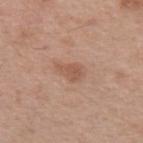Assessment: Captured during whole-body skin photography for melanoma surveillance; the lesion was not biopsied. Acquisition and patient details: Imaged with white-light lighting. A male subject, approximately 45 years of age. The lesion-visualizer software estimated a lesion area of about 4 mm², an eccentricity of roughly 0.75, and a symmetry-axis asymmetry near 0.4. It also reported a lesion color around L≈54 a*≈21 b*≈29 in CIELAB, about 8 CIELAB-L* units darker than the surrounding skin, and a normalized lesion–skin contrast near 6. The software also gave border irregularity of about 4 on a 0–10 scale and radial color variation of about 0.5. The software also gave an automated nevus-likeness rating near 10 out of 100. From the arm. A region of skin cropped from a whole-body photographic capture, roughly 15 mm wide.